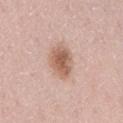Q: Is there a histopathology result?
A: imaged on a skin check; not biopsied
Q: What is the anatomic site?
A: the lower back
Q: How was this image acquired?
A: 15 mm crop, total-body photography
Q: What did automated image analysis measure?
A: an area of roughly 9.5 mm² and two-axis asymmetry of about 0.15; a border-irregularity rating of about 1.5/10, internal color variation of about 4 on a 0–10 scale, and a peripheral color-asymmetry measure near 1; lesion-presence confidence of about 100/100
Q: How was the tile lit?
A: white-light illumination
Q: Patient demographics?
A: female, in their 40s